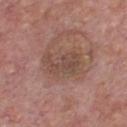This lesion was catalogued during total-body skin photography and was not selected for biopsy. The lesion is on the chest. A region of skin cropped from a whole-body photographic capture, roughly 15 mm wide. The tile uses white-light illumination. A male subject, in their mid- to late 70s. Longest diameter approximately 4.5 mm.